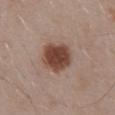  biopsy_status: not biopsied; imaged during a skin examination
  lesion_size:
    long_diameter_mm_approx: 4.5
  automated_metrics:
    area_mm2_approx: 13.0
    eccentricity: 0.55
    shape_asymmetry: 0.2
  image:
    source: total-body photography crop
    field_of_view_mm: 15
  site: mid back
  lighting: white-light
  patient:
    sex: male
    age_approx: 55